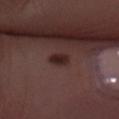The lesion-visualizer software estimated a lesion area of about 4 mm², an eccentricity of roughly 0.75, and a shape-asymmetry score of about 0.15 (0 = symmetric). The analysis additionally found a mean CIELAB color near L≈25 a*≈18 b*≈18 and a normalized border contrast of about 10.
Approximately 2.5 mm at its widest.
The patient is a female aged 48 to 52.
A 15 mm close-up extracted from a 3D total-body photography capture.
Located on the right forearm.